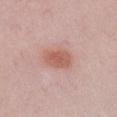subject: male, aged 48–52 | tile lighting: white-light illumination | site: the right upper arm | image-analysis metrics: a shape eccentricity near 0.75 and a shape-asymmetry score of about 0.15 (0 = symmetric); a border-irregularity rating of about 1.5/10, internal color variation of about 2.5 on a 0–10 scale, and peripheral color asymmetry of about 0.5 | image source: ~15 mm crop, total-body skin-cancer survey.